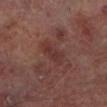Clinical impression:
The lesion was tiled from a total-body skin photograph and was not biopsied.
Image and clinical context:
Cropped from a whole-body photographic skin survey; the tile spans about 15 mm. Captured under cross-polarized illumination. The lesion is located on the left lower leg. A male subject about 65 years old.